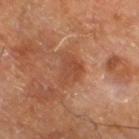From the right leg.
A male patient aged around 60.
A 15 mm close-up tile from a total-body photography series done for melanoma screening.
The tile uses cross-polarized illumination.